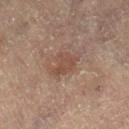Assessment:
The lesion was tiled from a total-body skin photograph and was not biopsied.
Context:
A lesion tile, about 15 mm wide, cut from a 3D total-body photograph. Automated tile analysis of the lesion measured an area of roughly 6.5 mm², a shape eccentricity near 0.75, and a symmetry-axis asymmetry near 0.35. And it measured a classifier nevus-likeness of about 5/100 and a lesion-detection confidence of about 100/100. A female patient aged around 80. The lesion is located on the leg. Measured at roughly 3.5 mm in maximum diameter. The tile uses cross-polarized illumination.